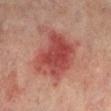| key | value |
|---|---|
| follow-up | imaged on a skin check; not biopsied |
| anatomic site | the left lower leg |
| subject | male, in their mid-70s |
| imaging modality | 15 mm crop, total-body photography |